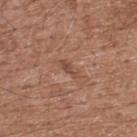No biopsy was performed on this lesion — it was imaged during a full skin examination and was not determined to be concerning. About 2.5 mm across. Automated tile analysis of the lesion measured a shape eccentricity near 0.9 and a symmetry-axis asymmetry near 0.4. The analysis additionally found a classifier nevus-likeness of about 0/100 and lesion-presence confidence of about 95/100. The tile uses white-light illumination. A male patient about 75 years old. From the back. This image is a 15 mm lesion crop taken from a total-body photograph.